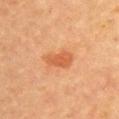Assessment:
No biopsy was performed on this lesion — it was imaged during a full skin examination and was not determined to be concerning.
Clinical summary:
A close-up tile cropped from a whole-body skin photograph, about 15 mm across. The lesion's longest dimension is about 4 mm. The lesion is on the upper back. A female patient, approximately 60 years of age. Captured under cross-polarized illumination. An algorithmic analysis of the crop reported an eccentricity of roughly 0.85 and two-axis asymmetry of about 0.25. It also reported a border-irregularity rating of about 2.5/10 and radial color variation of about 0.5.